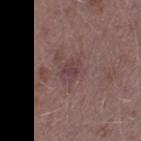Recorded during total-body skin imaging; not selected for excision or biopsy. From the left thigh. A male subject aged 38–42. Longest diameter approximately 3 mm. The lesion-visualizer software estimated a lesion area of about 5.5 mm², an outline eccentricity of about 0.6 (0 = round, 1 = elongated), and a symmetry-axis asymmetry near 0.25. And it measured a classifier nevus-likeness of about 0/100 and a lesion-detection confidence of about 100/100. Cropped from a whole-body photographic skin survey; the tile spans about 15 mm.